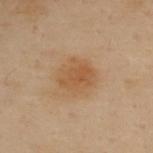lighting: cross-polarized
patient:
  sex: female
  age_approx: 60
lesion_size:
  long_diameter_mm_approx: 4.0
site: upper back
image:
  source: total-body photography crop
  field_of_view_mm: 15